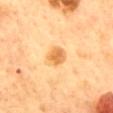Recorded during total-body skin imaging; not selected for excision or biopsy.
On the mid back.
The tile uses cross-polarized illumination.
Automated tile analysis of the lesion measured a lesion color around L≈63 a*≈22 b*≈45 in CIELAB and a lesion–skin lightness drop of about 10. The analysis additionally found border irregularity of about 1.5 on a 0–10 scale and peripheral color asymmetry of about 1. The software also gave a lesion-detection confidence of about 100/100.
The lesion's longest dimension is about 3 mm.
The patient is a female aged 58–62.
A 15 mm close-up extracted from a 3D total-body photography capture.